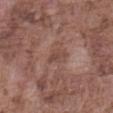Findings:
* notes · no biopsy performed (imaged during a skin exam)
* acquisition · 15 mm crop, total-body photography
* anatomic site · the abdomen
* lesion diameter · ≈2.5 mm
* subject · male, in their mid-70s
* illumination · white-light illumination